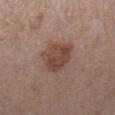Part of a total-body skin-imaging series; this lesion was reviewed on a skin check and was not flagged for biopsy. Located on the right lower leg. A region of skin cropped from a whole-body photographic capture, roughly 15 mm wide. The subject is a female aged 38 to 42.